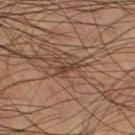biopsy_status: not biopsied; imaged during a skin examination
patient:
  sex: male
  age_approx: 60
site: left thigh
image:
  source: total-body photography crop
  field_of_view_mm: 15
automated_metrics:
  eccentricity: 0.9
  border_irregularity_0_10: 3.0
  color_variation_0_10: 0.0
  peripheral_color_asymmetry: 0.0
lesion_size:
  long_diameter_mm_approx: 2.5
lighting: cross-polarized